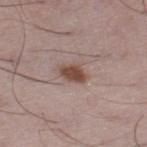biopsy_status: not biopsied; imaged during a skin examination
lesion_size:
  long_diameter_mm_approx: 3.0
automated_metrics:
  cielab_L: 47
  cielab_a: 19
  cielab_b: 24
  vs_skin_darker_L: 13.0
  border_irregularity_0_10: 2.5
  color_variation_0_10: 2.5
  peripheral_color_asymmetry: 1.0
  nevus_likeness_0_100: 95
  lesion_detection_confidence_0_100: 100
lighting: white-light
patient:
  sex: male
  age_approx: 50
image:
  source: total-body photography crop
  field_of_view_mm: 15
site: left thigh